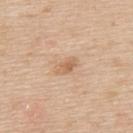Q: Is there a histopathology result?
A: no biopsy performed (imaged during a skin exam)
Q: What is the anatomic site?
A: the upper back
Q: How was the tile lit?
A: white-light illumination
Q: What is the lesion's diameter?
A: about 3 mm
Q: What is the imaging modality?
A: 15 mm crop, total-body photography
Q: What are the patient's age and sex?
A: male, aged 63 to 67
Q: Automated lesion metrics?
A: an area of roughly 4 mm², a shape eccentricity near 0.85, and a symmetry-axis asymmetry near 0.25; a mean CIELAB color near L≈63 a*≈19 b*≈35